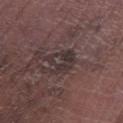Impression:
The lesion was tiled from a total-body skin photograph and was not biopsied.
Acquisition and patient details:
The lesion is on the left lower leg. A male subject roughly 75 years of age. This is a white-light tile. The recorded lesion diameter is about 4 mm. This image is a 15 mm lesion crop taken from a total-body photograph. An algorithmic analysis of the crop reported an area of roughly 8.5 mm² and a shape eccentricity near 0.6. It also reported a nevus-likeness score of about 0/100 and lesion-presence confidence of about 55/100.